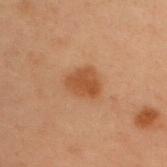• notes: imaged on a skin check; not biopsied
• acquisition: ~15 mm crop, total-body skin-cancer survey
• TBP lesion metrics: a color-variation rating of about 2.5/10 and a peripheral color-asymmetry measure near 0.5
• subject: male, aged 53–57
• location: the upper back
• lighting: cross-polarized